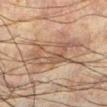| field | value |
|---|---|
| notes | imaged on a skin check; not biopsied |
| acquisition | ~15 mm tile from a whole-body skin photo |
| automated metrics | a footprint of about 16 mm² and a shape eccentricity near 0.75; roughly 7 lightness units darker than nearby skin and a normalized border contrast of about 6 |
| diameter | about 6 mm |
| patient | male, approximately 60 years of age |
| body site | the left lower leg |